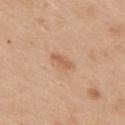Recorded during total-body skin imaging; not selected for excision or biopsy. Located on the back. Imaged with white-light lighting. The subject is a female aged 38 to 42. Approximately 3 mm at its widest. Automated tile analysis of the lesion measured a border-irregularity index near 3/10 and internal color variation of about 0.5 on a 0–10 scale. A roughly 15 mm field-of-view crop from a total-body skin photograph.